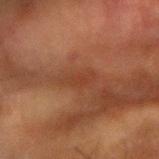Recorded during total-body skin imaging; not selected for excision or biopsy. This is a cross-polarized tile. The patient is a male aged 63–67. Longest diameter approximately 3.5 mm. Located on the left forearm. Cropped from a whole-body photographic skin survey; the tile spans about 15 mm.